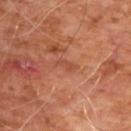<record>
  <biopsy_status>not biopsied; imaged during a skin examination</biopsy_status>
  <patient>
    <sex>male</sex>
    <age_approx>60</age_approx>
  </patient>
  <site>chest</site>
  <automated_metrics>
    <cielab_L>47</cielab_L>
    <cielab_a>27</cielab_a>
    <cielab_b>33</cielab_b>
    <vs_skin_darker_L>6.0</vs_skin_darker_L>
    <vs_skin_contrast_norm>5.0</vs_skin_contrast_norm>
    <color_variation_0_10>0.0</color_variation_0_10>
    <peripheral_color_asymmetry>0.0</peripheral_color_asymmetry>
    <lesion_detection_confidence_0_100>100</lesion_detection_confidence_0_100>
  </automated_metrics>
  <image>
    <source>total-body photography crop</source>
    <field_of_view_mm>15</field_of_view_mm>
  </image>
  <lighting>cross-polarized</lighting>
</record>